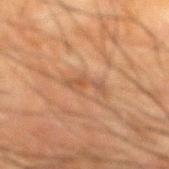Image and clinical context:
A 15 mm crop from a total-body photograph taken for skin-cancer surveillance. Located on the mid back. The subject is a male about 65 years old.